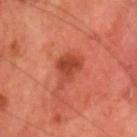Captured during whole-body skin photography for melanoma surveillance; the lesion was not biopsied. The lesion is located on the head or neck. The lesion-visualizer software estimated a footprint of about 7.5 mm², an outline eccentricity of about 0.7 (0 = round, 1 = elongated), and a symmetry-axis asymmetry near 0.4. It also reported a lesion color around L≈45 a*≈33 b*≈35 in CIELAB, about 9 CIELAB-L* units darker than the surrounding skin, and a lesion-to-skin contrast of about 7 (normalized; higher = more distinct). The software also gave border irregularity of about 4.5 on a 0–10 scale, a within-lesion color-variation index near 2.5/10, and radial color variation of about 1. It also reported a nevus-likeness score of about 75/100 and a detector confidence of about 100 out of 100 that the crop contains a lesion. A male subject aged around 70. Captured under cross-polarized illumination. A 15 mm crop from a total-body photograph taken for skin-cancer surveillance.